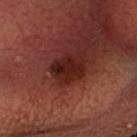Located on the head or neck. Captured under cross-polarized illumination. A male subject, aged 53–57. An algorithmic analysis of the crop reported a lesion color around L≈24 a*≈26 b*≈25 in CIELAB and about 10 CIELAB-L* units darker than the surrounding skin. A lesion tile, about 15 mm wide, cut from a 3D total-body photograph. Histopathologically confirmed as a solar lentigo.